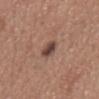Case summary:
* biopsy status · total-body-photography surveillance lesion; no biopsy
* image source · total-body-photography crop, ~15 mm field of view
* location · the abdomen
* tile lighting · white-light
* automated metrics · a nevus-likeness score of about 80/100 and a lesion-detection confidence of about 100/100
* subject · male, in their mid-60s